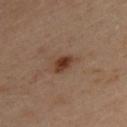No biopsy was performed on this lesion — it was imaged during a full skin examination and was not determined to be concerning.
A close-up tile cropped from a whole-body skin photograph, about 15 mm across.
A male patient in their mid-30s.
From the upper back.